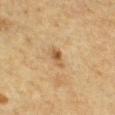The lesion was photographed on a routine skin check and not biopsied; there is no pathology result. The subject is a male in their mid- to late 60s. The lesion-visualizer software estimated a footprint of about 3 mm², an outline eccentricity of about 0.85 (0 = round, 1 = elongated), and two-axis asymmetry of about 0.35. And it measured border irregularity of about 3.5 on a 0–10 scale and a within-lesion color-variation index near 2/10. And it measured a detector confidence of about 100 out of 100 that the crop contains a lesion. Cropped from a whole-body photographic skin survey; the tile spans about 15 mm. Captured under cross-polarized illumination. On the front of the torso.